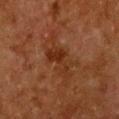| field | value |
|---|---|
| workup | imaged on a skin check; not biopsied |
| patient | female, aged around 50 |
| lesion size | ~6.5 mm (longest diameter) |
| site | the chest |
| image source | 15 mm crop, total-body photography |